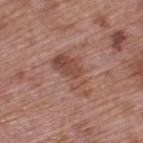| key | value |
|---|---|
| imaging modality | ~15 mm crop, total-body skin-cancer survey |
| location | the upper back |
| diameter | about 6 mm |
| illumination | white-light |
| subject | male, in their 70s |